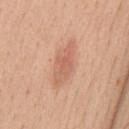Notes:
- anatomic site — the mid back
- diameter — ≈5.5 mm
- patient — male, aged approximately 40
- illumination — white-light illumination
- imaging modality — ~15 mm tile from a whole-body skin photo